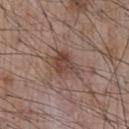follow-up: no biopsy performed (imaged during a skin exam) | diameter: about 3.5 mm | anatomic site: the chest | image source: total-body-photography crop, ~15 mm field of view | subject: male, in their mid-50s.